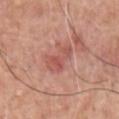| key | value |
|---|---|
| notes | total-body-photography surveillance lesion; no biopsy |
| lighting | white-light illumination |
| acquisition | 15 mm crop, total-body photography |
| anatomic site | the chest |
| lesion size | ~4 mm (longest diameter) |
| subject | male, about 60 years old |